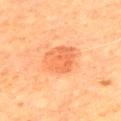A male subject, in their 80s. This is a cross-polarized tile. From the upper back. A region of skin cropped from a whole-body photographic capture, roughly 15 mm wide.